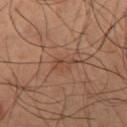Impression: Imaged during a routine full-body skin examination; the lesion was not biopsied and no histopathology is available. Clinical summary: From the right thigh. A male patient aged approximately 65. An algorithmic analysis of the crop reported an area of roughly 3 mm², an eccentricity of roughly 0.85, and two-axis asymmetry of about 0.5. And it measured a lesion color around L≈44 a*≈20 b*≈29 in CIELAB, about 6 CIELAB-L* units darker than the surrounding skin, and a normalized border contrast of about 5.5. It also reported a lesion-detection confidence of about 95/100. The tile uses cross-polarized illumination. A lesion tile, about 15 mm wide, cut from a 3D total-body photograph. About 2.5 mm across.